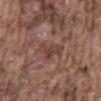Clinical summary:
The patient is a male aged around 75. A lesion tile, about 15 mm wide, cut from a 3D total-body photograph. From the mid back.